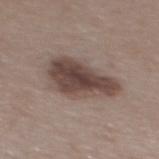A female subject aged around 45. Located on the mid back. This image is a 15 mm lesion crop taken from a total-body photograph.